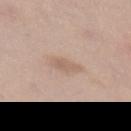The patient is a female aged 38 to 42. The total-body-photography lesion software estimated a nevus-likeness score of about 10/100. A 15 mm close-up extracted from a 3D total-body photography capture. The tile uses white-light illumination. Approximately 3 mm at its widest. The lesion is on the front of the torso.